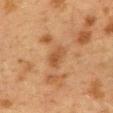follow-up: imaged on a skin check; not biopsied | location: the back | subject: female, approximately 40 years of age | image source: ~15 mm crop, total-body skin-cancer survey.